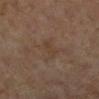Q: Was a biopsy performed?
A: imaged on a skin check; not biopsied
Q: What is the lesion's diameter?
A: ~3 mm (longest diameter)
Q: Patient demographics?
A: female, aged 58–62
Q: Lesion location?
A: the left lower leg
Q: How was this image acquired?
A: total-body-photography crop, ~15 mm field of view